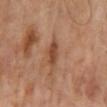follow-up = imaged on a skin check; not biopsied
image source = 15 mm crop, total-body photography
site = the mid back
patient = male, in their mid- to late 60s